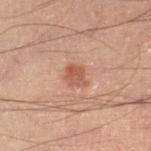Clinical impression: Captured during whole-body skin photography for melanoma surveillance; the lesion was not biopsied. Context: A male patient aged 48–52. On the right lower leg. Measured at roughly 2.5 mm in maximum diameter. A 15 mm crop from a total-body photograph taken for skin-cancer surveillance. The total-body-photography lesion software estimated a lesion color around L≈48 a*≈23 b*≈28 in CIELAB and a lesion–skin lightness drop of about 9. The software also gave a border-irregularity rating of about 2/10 and a within-lesion color-variation index near 3/10. The software also gave a lesion-detection confidence of about 100/100.